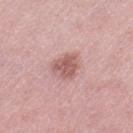The lesion was tiled from a total-body skin photograph and was not biopsied. A roughly 15 mm field-of-view crop from a total-body skin photograph. The subject is a female roughly 40 years of age. The lesion is located on the leg. This is a white-light tile. Approximately 3.5 mm at its widest.